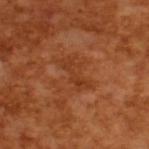This lesion was catalogued during total-body skin photography and was not selected for biopsy. This image is a 15 mm lesion crop taken from a total-body photograph. The tile uses cross-polarized illumination. The subject is a male approximately 65 years of age. Longest diameter approximately 4 mm.